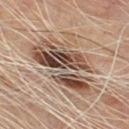Acquisition and patient details:
Cropped from a whole-body photographic skin survey; the tile spans about 15 mm. From the chest. A male subject aged 63–67. Captured under cross-polarized illumination.